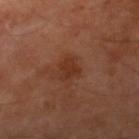The lesion was tiled from a total-body skin photograph and was not biopsied.
Cropped from a total-body skin-imaging series; the visible field is about 15 mm.
Approximately 2.5 mm at its widest.
A male subject, aged approximately 70.
Imaged with cross-polarized lighting.
The lesion is on the right lower leg.